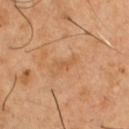The lesion was tiled from a total-body skin photograph and was not biopsied.
Approximately 3 mm at its widest.
A male subject aged approximately 50.
From the chest.
Cropped from a whole-body photographic skin survey; the tile spans about 15 mm.
The lesion-visualizer software estimated a shape-asymmetry score of about 0.5 (0 = symmetric). And it measured an average lesion color of about L≈56 a*≈22 b*≈39 (CIELAB) and about 6 CIELAB-L* units darker than the surrounding skin. The analysis additionally found a border-irregularity rating of about 5.5/10 and a color-variation rating of about 0/10. It also reported a classifier nevus-likeness of about 0/100 and a lesion-detection confidence of about 100/100.